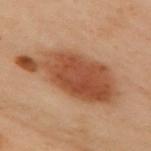Imaged during a routine full-body skin examination; the lesion was not biopsied and no histopathology is available.
A female subject, in their 60s.
Cropped from a total-body skin-imaging series; the visible field is about 15 mm.
On the back.
The lesion's longest dimension is about 10 mm.
The tile uses cross-polarized illumination.
The lesion-visualizer software estimated an area of roughly 37 mm². The analysis additionally found about 11 CIELAB-L* units darker than the surrounding skin.